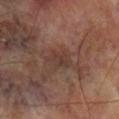Clinical impression: Captured during whole-body skin photography for melanoma surveillance; the lesion was not biopsied. Context: The lesion's longest dimension is about 3.5 mm. This image is a 15 mm lesion crop taken from a total-body photograph. A male subject, about 70 years old. Automated tile analysis of the lesion measured a lesion area of about 5.5 mm², an eccentricity of roughly 0.75, and a symmetry-axis asymmetry near 0.3. The lesion is located on the right lower leg. Imaged with cross-polarized lighting.